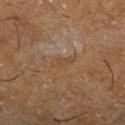biopsy status — catalogued during a skin exam; not biopsied